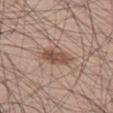No biopsy was performed on this lesion — it was imaged during a full skin examination and was not determined to be concerning. From the left thigh. A male patient, roughly 55 years of age. Captured under white-light illumination. A roughly 15 mm field-of-view crop from a total-body skin photograph.